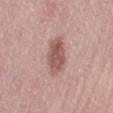Q: Was a biopsy performed?
A: catalogued during a skin exam; not biopsied
Q: What is the anatomic site?
A: the left thigh
Q: What are the patient's age and sex?
A: female, aged 63–67
Q: What lighting was used for the tile?
A: white-light illumination
Q: How was this image acquired?
A: ~15 mm tile from a whole-body skin photo